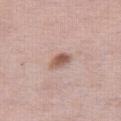biopsy status = catalogued during a skin exam; not biopsied | body site = the right thigh | tile lighting = white-light | image source = 15 mm crop, total-body photography | subject = female, aged 38 to 42 | automated metrics = border irregularity of about 2 on a 0–10 scale and a peripheral color-asymmetry measure near 1.5 | lesion diameter = about 3 mm.